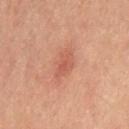Assessment:
Recorded during total-body skin imaging; not selected for excision or biopsy.
Acquisition and patient details:
A male subject, approximately 60 years of age. The lesion-visualizer software estimated a lesion area of about 5 mm² and a shape-asymmetry score of about 0.25 (0 = symmetric). The software also gave an average lesion color of about L≈42 a*≈21 b*≈25 (CIELAB), roughly 6 lightness units darker than nearby skin, and a normalized lesion–skin contrast near 5. The analysis additionally found a border-irregularity index near 3/10 and internal color variation of about 2 on a 0–10 scale. It also reported a classifier nevus-likeness of about 45/100 and a detector confidence of about 100 out of 100 that the crop contains a lesion. The lesion is located on the abdomen. The tile uses cross-polarized illumination. A 15 mm close-up extracted from a 3D total-body photography capture.